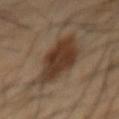notes = catalogued during a skin exam; not biopsied | site = the mid back | patient = male, approximately 45 years of age | imaging modality = 15 mm crop, total-body photography.